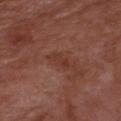Q: Was a biopsy performed?
A: imaged on a skin check; not biopsied
Q: Illumination type?
A: white-light
Q: How was this image acquired?
A: ~15 mm crop, total-body skin-cancer survey
Q: Who is the patient?
A: male, in their mid- to late 60s
Q: Where on the body is the lesion?
A: the front of the torso
Q: How large is the lesion?
A: about 3 mm
Q: What did automated image analysis measure?
A: an area of roughly 3.5 mm² and an outline eccentricity of about 0.9 (0 = round, 1 = elongated); a lesion color around L≈36 a*≈24 b*≈27 in CIELAB and a normalized border contrast of about 5.5; border irregularity of about 4 on a 0–10 scale and peripheral color asymmetry of about 0; a nevus-likeness score of about 0/100 and a lesion-detection confidence of about 100/100